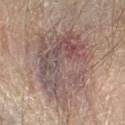Findings:
- workup · no biopsy performed (imaged during a skin exam)
- anatomic site · the arm
- patient · male, approximately 80 years of age
- lighting · white-light illumination
- acquisition · ~15 mm crop, total-body skin-cancer survey
- lesion diameter · ≈9 mm